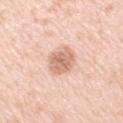Assessment: Captured during whole-body skin photography for melanoma surveillance; the lesion was not biopsied.